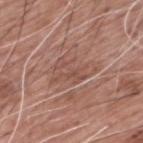  biopsy_status: not biopsied; imaged during a skin examination